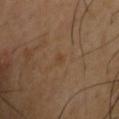  biopsy_status: not biopsied; imaged during a skin examination